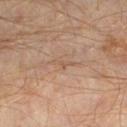follow-up: total-body-photography surveillance lesion; no biopsy | TBP lesion metrics: a lesion color around L≈51 a*≈16 b*≈28 in CIELAB, about 6 CIELAB-L* units darker than the surrounding skin, and a normalized lesion–skin contrast near 5; a border-irregularity index near 7/10, a within-lesion color-variation index near 0/10, and a peripheral color-asymmetry measure near 0; lesion-presence confidence of about 80/100 | illumination: cross-polarized illumination | location: the left lower leg | imaging modality: 15 mm crop, total-body photography | diameter: ≈2.5 mm | patient: male, in their 60s.